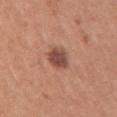The lesion was photographed on a routine skin check and not biopsied; there is no pathology result. The recorded lesion diameter is about 3.5 mm. Cropped from a total-body skin-imaging series; the visible field is about 15 mm. A female subject about 30 years old. On the right upper arm.